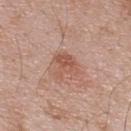Q: Was a biopsy performed?
A: imaged on a skin check; not biopsied
Q: What did automated image analysis measure?
A: an average lesion color of about L≈55 a*≈22 b*≈29 (CIELAB) and roughly 9 lightness units darker than nearby skin; a border-irregularity index near 4/10, internal color variation of about 4 on a 0–10 scale, and radial color variation of about 1.5; a classifier nevus-likeness of about 10/100 and a detector confidence of about 100 out of 100 that the crop contains a lesion
Q: Patient demographics?
A: male, aged 68 to 72
Q: How large is the lesion?
A: ~3 mm (longest diameter)
Q: What kind of image is this?
A: ~15 mm tile from a whole-body skin photo
Q: Where on the body is the lesion?
A: the upper back